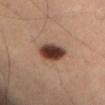This lesion was catalogued during total-body skin photography and was not selected for biopsy.
Located on the left thigh.
Imaged with cross-polarized lighting.
A 15 mm close-up tile from a total-body photography series done for melanoma screening.
The subject is a male about 55 years old.
Approximately 4 mm at its widest.
The total-body-photography lesion software estimated a shape-asymmetry score of about 0.2 (0 = symmetric). And it measured a lesion color around L≈37 a*≈19 b*≈25 in CIELAB, a lesion–skin lightness drop of about 18, and a normalized border contrast of about 14. The analysis additionally found border irregularity of about 2 on a 0–10 scale, a within-lesion color-variation index near 6/10, and radial color variation of about 1.5. It also reported lesion-presence confidence of about 100/100.